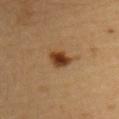Impression: Imaged during a routine full-body skin examination; the lesion was not biopsied and no histopathology is available. Image and clinical context: On the left upper arm. A female subject, in their 30s. This image is a 15 mm lesion crop taken from a total-body photograph. Captured under cross-polarized illumination. About 3 mm across.